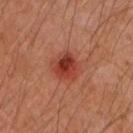workup: catalogued during a skin exam; not biopsied